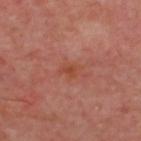workup = total-body-photography surveillance lesion; no biopsy
lesion size = ≈2.5 mm
site = the upper back
lighting = cross-polarized
automated metrics = a footprint of about 4 mm², a shape eccentricity near 0.65, and a symmetry-axis asymmetry near 0.25; a border-irregularity rating of about 2.5/10 and radial color variation of about 0.5; a classifier nevus-likeness of about 0/100 and lesion-presence confidence of about 100/100
image source = ~15 mm crop, total-body skin-cancer survey
subject = aged 53 to 57